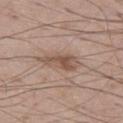lesion diameter=≈4.5 mm; patient=male, in their 50s; image=total-body-photography crop, ~15 mm field of view; site=the left thigh.